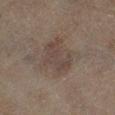Case summary:
- notes: no biopsy performed (imaged during a skin exam)
- site: the right lower leg
- subject: female, about 60 years old
- image: ~15 mm tile from a whole-body skin photo